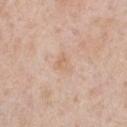The lesion was tiled from a total-body skin photograph and was not biopsied.
The patient is a male aged 48 to 52.
From the chest.
About 2.5 mm across.
A lesion tile, about 15 mm wide, cut from a 3D total-body photograph.